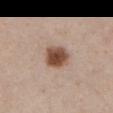notes = catalogued during a skin exam; not biopsied.